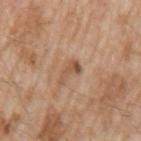Findings:
– follow-up · no biopsy performed (imaged during a skin exam)
– acquisition · ~15 mm tile from a whole-body skin photo
– diameter · about 3 mm
– lighting · white-light illumination
– subject · male, approximately 65 years of age
– anatomic site · the left upper arm